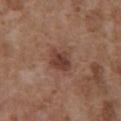Impression:
The lesion was photographed on a routine skin check and not biopsied; there is no pathology result.
Background:
A male patient aged 73 to 77. Measured at roughly 3 mm in maximum diameter. The lesion is located on the abdomen. A 15 mm close-up extracted from a 3D total-body photography capture. This is a white-light tile.